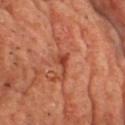The lesion was photographed on a routine skin check and not biopsied; there is no pathology result. Measured at roughly 3 mm in maximum diameter. The lesion is located on the front of the torso. A 15 mm close-up extracted from a 3D total-body photography capture. An algorithmic analysis of the crop reported a border-irregularity index near 5.5/10 and a peripheral color-asymmetry measure near 1. It also reported a classifier nevus-likeness of about 0/100 and a detector confidence of about 100 out of 100 that the crop contains a lesion. This is a cross-polarized tile. A male subject about 65 years old.